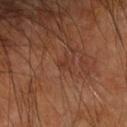follow-up = no biopsy performed (imaged during a skin exam)
subject = male, aged 58–62
anatomic site = the arm
tile lighting = cross-polarized
imaging modality = ~15 mm tile from a whole-body skin photo